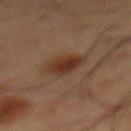– biopsy status · imaged on a skin check; not biopsied
– patient · male, aged around 60
– lesion diameter · ≈3 mm
– lighting · cross-polarized illumination
– automated lesion analysis · a footprint of about 7 mm², an outline eccentricity of about 0.5 (0 = round, 1 = elongated), and a shape-asymmetry score of about 0.2 (0 = symmetric); an automated nevus-likeness rating near 95 out of 100
– acquisition · ~15 mm crop, total-body skin-cancer survey
– anatomic site · the mid back